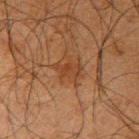Image and clinical context: Imaged with cross-polarized lighting. The subject is a male aged approximately 65. Approximately 3.5 mm at its widest. A 15 mm crop from a total-body photograph taken for skin-cancer surveillance. The lesion is on the arm.